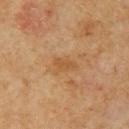Impression:
Recorded during total-body skin imaging; not selected for excision or biopsy.
Image and clinical context:
Located on the left upper arm. A 15 mm close-up tile from a total-body photography series done for melanoma screening. A male subject roughly 60 years of age. About 2.5 mm across.